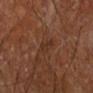Assessment: The lesion was tiled from a total-body skin photograph and was not biopsied. Acquisition and patient details: The tile uses cross-polarized illumination. Located on the right forearm. Approximately 3 mm at its widest. A male subject, aged approximately 70. A 15 mm close-up extracted from a 3D total-body photography capture.